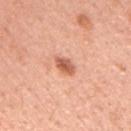Assessment:
Captured during whole-body skin photography for melanoma surveillance; the lesion was not biopsied.
Background:
A male subject aged approximately 65. This image is a 15 mm lesion crop taken from a total-body photograph. On the mid back.